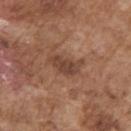This is a white-light tile.
The lesion-visualizer software estimated a nevus-likeness score of about 45/100 and lesion-presence confidence of about 100/100.
The recorded lesion diameter is about 4 mm.
A male subject, approximately 75 years of age.
Located on the right upper arm.
A lesion tile, about 15 mm wide, cut from a 3D total-body photograph.